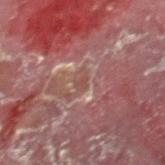Image and clinical context: A male subject, approximately 55 years of age. The tile uses cross-polarized illumination. On the leg. Cropped from a whole-body photographic skin survey; the tile spans about 15 mm. The lesion's longest dimension is about 1.5 mm.